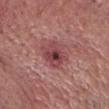- workup · total-body-photography surveillance lesion; no biopsy
- subject · male, aged around 75
- image · 15 mm crop, total-body photography
- lighting · white-light illumination
- anatomic site · the front of the torso